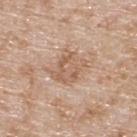biopsy status = catalogued during a skin exam; not biopsied | acquisition = ~15 mm tile from a whole-body skin photo | subject = male, in their 80s | body site = the upper back | lesion diameter = ~4.5 mm (longest diameter) | automated metrics = a footprint of about 12 mm² and an eccentricity of roughly 0.65; lesion-presence confidence of about 100/100.